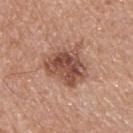Captured during whole-body skin photography for melanoma surveillance; the lesion was not biopsied.